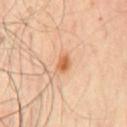This lesion was catalogued during total-body skin photography and was not selected for biopsy. Cropped from a total-body skin-imaging series; the visible field is about 15 mm. Approximately 2.5 mm at its widest. Captured under cross-polarized illumination. The subject is a male aged 63–67. From the chest.